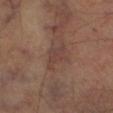Captured during whole-body skin photography for melanoma surveillance; the lesion was not biopsied.
Imaged with cross-polarized lighting.
Longest diameter approximately 3.5 mm.
The patient is a male aged 58 to 62.
A lesion tile, about 15 mm wide, cut from a 3D total-body photograph.
Automated image analysis of the tile measured a lesion area of about 5 mm², an outline eccentricity of about 0.8 (0 = round, 1 = elongated), and two-axis asymmetry of about 0.45. And it measured a lesion color around L≈38 a*≈18 b*≈22 in CIELAB, roughly 6 lightness units darker than nearby skin, and a lesion-to-skin contrast of about 5.5 (normalized; higher = more distinct). It also reported border irregularity of about 5 on a 0–10 scale, internal color variation of about 2.5 on a 0–10 scale, and radial color variation of about 1.
The lesion is located on the leg.